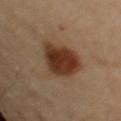Recorded during total-body skin imaging; not selected for excision or biopsy.
This image is a 15 mm lesion crop taken from a total-body photograph.
The tile uses cross-polarized illumination.
Located on the right arm.
A female patient about 60 years old.